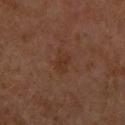<lesion>
  <biopsy_status>not biopsied; imaged during a skin examination</biopsy_status>
  <site>right upper arm</site>
  <patient>
    <sex>female</sex>
    <age_approx>60</age_approx>
  </patient>
  <lighting>cross-polarized</lighting>
  <image>
    <source>total-body photography crop</source>
    <field_of_view_mm>15</field_of_view_mm>
  </image>
  <lesion_size>
    <long_diameter_mm_approx>3.0</long_diameter_mm_approx>
  </lesion_size>
</lesion>